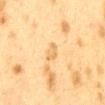{
  "biopsy_status": "not biopsied; imaged during a skin examination",
  "lesion_size": {
    "long_diameter_mm_approx": 3.0
  },
  "lighting": "cross-polarized",
  "image": {
    "source": "total-body photography crop",
    "field_of_view_mm": 15
  },
  "site": "mid back",
  "patient": {
    "sex": "female",
    "age_approx": 40
  }
}